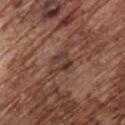Impression:
Imaged during a routine full-body skin examination; the lesion was not biopsied and no histopathology is available.
Background:
A lesion tile, about 15 mm wide, cut from a 3D total-body photograph. From the chest. Approximately 2.5 mm at its widest. An algorithmic analysis of the crop reported a border-irregularity index near 3.5/10, internal color variation of about 8.5 on a 0–10 scale, and peripheral color asymmetry of about 2.5. It also reported a classifier nevus-likeness of about 0/100 and a lesion-detection confidence of about 95/100. A male patient in their mid- to late 70s. This is a white-light tile.